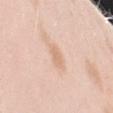No biopsy was performed on this lesion — it was imaged during a full skin examination and was not determined to be concerning.
A region of skin cropped from a whole-body photographic capture, roughly 15 mm wide.
Approximately 2.5 mm at its widest.
Located on the left upper arm.
A female patient approximately 25 years of age.
Imaged with white-light lighting.